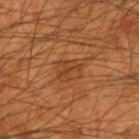The lesion was tiled from a total-body skin photograph and was not biopsied.
On the left forearm.
A 15 mm crop from a total-body photograph taken for skin-cancer surveillance.
Approximately 3.5 mm at its widest.
The patient is a male approximately 55 years of age.